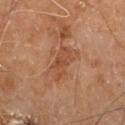biopsy_status: not biopsied; imaged during a skin examination
image:
  source: total-body photography crop
  field_of_view_mm: 15
patient:
  sex: male
  age_approx: 60
lighting: cross-polarized
site: right leg